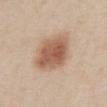Q: How was the tile lit?
A: white-light illumination
Q: What are the patient's age and sex?
A: male, in their 60s
Q: What is the imaging modality?
A: 15 mm crop, total-body photography
Q: How large is the lesion?
A: ≈5 mm
Q: Where on the body is the lesion?
A: the abdomen